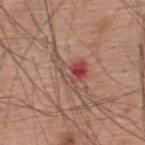The lesion is on the upper back.
This image is a 15 mm lesion crop taken from a total-body photograph.
A male subject aged around 60.
This is a white-light tile.
The total-body-photography lesion software estimated border irregularity of about 6 on a 0–10 scale and internal color variation of about 10 on a 0–10 scale. It also reported an automated nevus-likeness rating near 0 out of 100.
About 4 mm across.